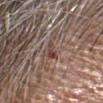follow-up: no biopsy performed (imaged during a skin exam) | lesion diameter: ≈3 mm | image source: ~15 mm crop, total-body skin-cancer survey | tile lighting: white-light illumination | image-analysis metrics: a shape eccentricity near 0.85 and a symmetry-axis asymmetry near 0.4; a mean CIELAB color near L≈43 a*≈18 b*≈20 and roughly 9 lightness units darker than nearby skin; a border-irregularity rating of about 4/10 and internal color variation of about 2.5 on a 0–10 scale; a nevus-likeness score of about 0/100 and a lesion-detection confidence of about 85/100 | body site: the head or neck | patient: male, about 60 years old.